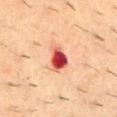Notes:
– notes: imaged on a skin check; not biopsied
– acquisition: ~15 mm crop, total-body skin-cancer survey
– TBP lesion metrics: an outline eccentricity of about 0.75 (0 = round, 1 = elongated) and a shape-asymmetry score of about 0.35 (0 = symmetric); an average lesion color of about L≈47 a*≈36 b*≈30 (CIELAB), roughly 20 lightness units darker than nearby skin, and a lesion-to-skin contrast of about 13.5 (normalized; higher = more distinct); a border-irregularity rating of about 3.5/10, a color-variation rating of about 8.5/10, and a peripheral color-asymmetry measure near 2.5
– patient: male, approximately 55 years of age
– size: about 3.5 mm
– body site: the mid back
– lighting: cross-polarized illumination